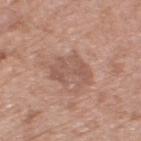Q: Was this lesion biopsied?
A: imaged on a skin check; not biopsied
Q: What are the patient's age and sex?
A: female, roughly 75 years of age
Q: How was this image acquired?
A: ~15 mm crop, total-body skin-cancer survey
Q: What is the anatomic site?
A: the left thigh